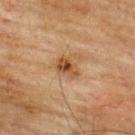This lesion was catalogued during total-body skin photography and was not selected for biopsy. About 3 mm across. A male subject, aged 73–77. This is a cross-polarized tile. A 15 mm close-up extracted from a 3D total-body photography capture. The lesion is located on the upper back.